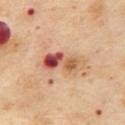Assessment:
No biopsy was performed on this lesion — it was imaged during a full skin examination and was not determined to be concerning.
Acquisition and patient details:
Located on the mid back. A female patient about 55 years old. About 5.5 mm across. Automated tile analysis of the lesion measured a footprint of about 11 mm², an outline eccentricity of about 0.9 (0 = round, 1 = elongated), and a shape-asymmetry score of about 0.25 (0 = symmetric). It also reported border irregularity of about 3.5 on a 0–10 scale and radial color variation of about 6.5. It also reported an automated nevus-likeness rating near 0 out of 100. Imaged with cross-polarized lighting. A 15 mm crop from a total-body photograph taken for skin-cancer surveillance.